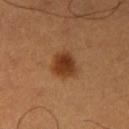Notes:
– follow-up · total-body-photography surveillance lesion; no biopsy
– lighting · cross-polarized illumination
– body site · the left thigh
– diameter · ~3.5 mm (longest diameter)
– acquisition · total-body-photography crop, ~15 mm field of view
– patient · male, roughly 50 years of age